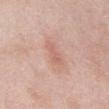| field | value |
|---|---|
| notes | no biopsy performed (imaged during a skin exam) |
| site | the abdomen |
| subject | male, in their mid-50s |
| TBP lesion metrics | a lesion area of about 4.5 mm², an eccentricity of roughly 0.9, and a shape-asymmetry score of about 0.35 (0 = symmetric); a mean CIELAB color near L≈63 a*≈23 b*≈27; border irregularity of about 4 on a 0–10 scale, internal color variation of about 1.5 on a 0–10 scale, and radial color variation of about 0.5 |
| acquisition | total-body-photography crop, ~15 mm field of view |